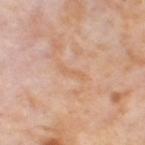{"biopsy_status": "not biopsied; imaged during a skin examination", "automated_metrics": {"cielab_L": 64, "cielab_a": 21, "cielab_b": 35, "vs_skin_darker_L": 6.0, "vs_skin_contrast_norm": 4.5}, "site": "leg", "patient": {"sex": "female", "age_approx": 55}, "lighting": "cross-polarized", "image": {"source": "total-body photography crop", "field_of_view_mm": 15}, "lesion_size": {"long_diameter_mm_approx": 3.0}}